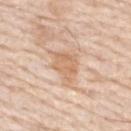follow-up=catalogued during a skin exam; not biopsied | patient=male, aged around 75 | anatomic site=the back | image source=~15 mm crop, total-body skin-cancer survey | lesion size=~4 mm (longest diameter) | illumination=white-light.